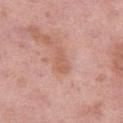• notes — total-body-photography surveillance lesion; no biopsy
• lighting — white-light illumination
• acquisition — ~15 mm tile from a whole-body skin photo
• subject — female, about 50 years old
• automated metrics — a border-irregularity rating of about 3/10, a color-variation rating of about 1.5/10, and peripheral color asymmetry of about 0.5; a nevus-likeness score of about 0/100 and a detector confidence of about 100 out of 100 that the crop contains a lesion
• location — the left thigh
• size — about 3.5 mm